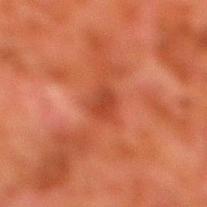- follow-up — catalogued during a skin exam; not biopsied
- subject — male, approximately 80 years of age
- diameter — ≈2.5 mm
- image — total-body-photography crop, ~15 mm field of view
- body site — the right lower leg